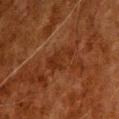<tbp_lesion>
<biopsy_status>not biopsied; imaged during a skin examination</biopsy_status>
<lesion_size>
  <long_diameter_mm_approx>4.0</long_diameter_mm_approx>
</lesion_size>
<patient>
  <sex>male</sex>
  <age_approx>80</age_approx>
</patient>
<site>head or neck</site>
<image>
  <source>total-body photography crop</source>
  <field_of_view_mm>15</field_of_view_mm>
</image>
<lighting>cross-polarized</lighting>
<automated_metrics>
  <area_mm2_approx>6.5</area_mm2_approx>
  <eccentricity>0.8</eccentricity>
  <shape_asymmetry>0.35</shape_asymmetry>
  <border_irregularity_0_10>5.5</border_irregularity_0_10>
  <nevus_likeness_0_100>0</nevus_likeness_0_100>
  <lesion_detection_confidence_0_100>95</lesion_detection_confidence_0_100>
</automated_metrics>
</tbp_lesion>